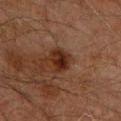biopsy status — total-body-photography surveillance lesion; no biopsy
lighting — cross-polarized illumination
automated metrics — a lesion area of about 4.5 mm², an eccentricity of roughly 0.7, and a symmetry-axis asymmetry near 0.2; border irregularity of about 2 on a 0–10 scale, a within-lesion color-variation index near 4.5/10, and peripheral color asymmetry of about 1.5
location — the chest
imaging modality — ~15 mm tile from a whole-body skin photo
subject — male, about 60 years old